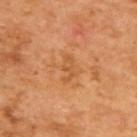Clinical impression: Imaged during a routine full-body skin examination; the lesion was not biopsied and no histopathology is available. Acquisition and patient details: Captured under cross-polarized illumination. From the upper back. The subject is a male aged approximately 65. A region of skin cropped from a whole-body photographic capture, roughly 15 mm wide.